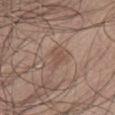<record>
<patient>
  <sex>male</sex>
  <age_approx>25</age_approx>
</patient>
<lighting>white-light</lighting>
<lesion_size>
  <long_diameter_mm_approx>2.5</long_diameter_mm_approx>
</lesion_size>
<site>abdomen</site>
<image>
  <source>total-body photography crop</source>
  <field_of_view_mm>15</field_of_view_mm>
</image>
</record>